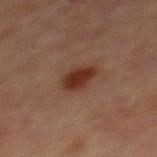Assessment: No biopsy was performed on this lesion — it was imaged during a full skin examination and was not determined to be concerning. Clinical summary: The lesion is on the chest. Cropped from a total-body skin-imaging series; the visible field is about 15 mm. The subject is a male in their mid- to late 60s.